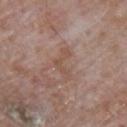• location · the back
• patient · male, aged around 65
• size · about 4 mm
• image · ~15 mm crop, total-body skin-cancer survey